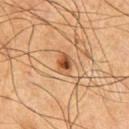No biopsy was performed on this lesion — it was imaged during a full skin examination and was not determined to be concerning.
A male subject, aged 63–67.
On the chest.
Cropped from a whole-body photographic skin survey; the tile spans about 15 mm.